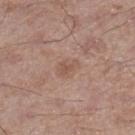Assessment:
Recorded during total-body skin imaging; not selected for excision or biopsy.
Context:
The tile uses white-light illumination. An algorithmic analysis of the crop reported a border-irregularity rating of about 4/10, a color-variation rating of about 0.5/10, and a peripheral color-asymmetry measure near 0. And it measured lesion-presence confidence of about 100/100. Located on the right lower leg. A 15 mm close-up extracted from a 3D total-body photography capture. A male subject, aged around 55. Measured at roughly 2.5 mm in maximum diameter.